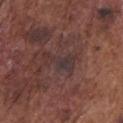Impression:
The lesion was tiled from a total-body skin photograph and was not biopsied.
Clinical summary:
The lesion's longest dimension is about 4.5 mm. A male subject roughly 75 years of age. A region of skin cropped from a whole-body photographic capture, roughly 15 mm wide. From the chest. The total-body-photography lesion software estimated a footprint of about 6 mm², an outline eccentricity of about 0.9 (0 = round, 1 = elongated), and two-axis asymmetry of about 0.6. The analysis additionally found a mean CIELAB color near L≈33 a*≈16 b*≈16 and a lesion–skin lightness drop of about 6.